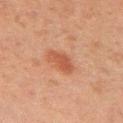Findings:
- follow-up: imaged on a skin check; not biopsied